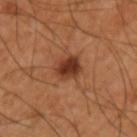Recorded during total-body skin imaging; not selected for excision or biopsy. Cropped from a whole-body photographic skin survey; the tile spans about 15 mm. Longest diameter approximately 3 mm. An algorithmic analysis of the crop reported a shape eccentricity near 0.55 and a symmetry-axis asymmetry near 0.2. This is a cross-polarized tile. The patient is a male in their mid-60s. The lesion is located on the left upper arm.